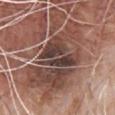follow-up: total-body-photography surveillance lesion; no biopsy
diameter: about 12 mm
tile lighting: white-light illumination
anatomic site: the front of the torso
acquisition: ~15 mm tile from a whole-body skin photo
image-analysis metrics: an area of roughly 55 mm², an outline eccentricity of about 0.8 (0 = round, 1 = elongated), and a symmetry-axis asymmetry near 0.4; a border-irregularity rating of about 8/10 and a within-lesion color-variation index near 8.5/10
subject: male, aged 63–67